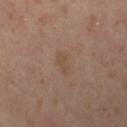{
  "biopsy_status": "not biopsied; imaged during a skin examination",
  "site": "left thigh",
  "lighting": "cross-polarized",
  "patient": {
    "sex": "female",
    "age_approx": 40
  },
  "lesion_size": {
    "long_diameter_mm_approx": 3.0
  },
  "image": {
    "source": "total-body photography crop",
    "field_of_view_mm": 15
  }
}